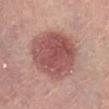notes: total-body-photography surveillance lesion; no biopsy
illumination: white-light illumination
site: the abdomen
diameter: about 7 mm
automated metrics: a nevus-likeness score of about 95/100 and a detector confidence of about 100 out of 100 that the crop contains a lesion
subject: female, in their mid- to late 60s
acquisition: total-body-photography crop, ~15 mm field of view